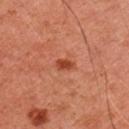{
  "lesion_size": {
    "long_diameter_mm_approx": 2.0
  },
  "site": "right upper arm",
  "patient": {
    "sex": "male",
    "age_approx": 45
  },
  "lighting": "cross-polarized",
  "image": {
    "source": "total-body photography crop",
    "field_of_view_mm": 15
  }
}